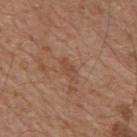Part of a total-body skin-imaging series; this lesion was reviewed on a skin check and was not flagged for biopsy. Measured at roughly 2.5 mm in maximum diameter. The subject is a male aged 63 to 67. A close-up tile cropped from a whole-body skin photograph, about 15 mm across. Located on the mid back.